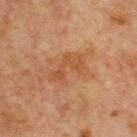This lesion was catalogued during total-body skin photography and was not selected for biopsy.
A male patient, about 65 years old.
Cropped from a total-body skin-imaging series; the visible field is about 15 mm.
The lesion's longest dimension is about 4.5 mm.
Captured under cross-polarized illumination.
From the chest.
An algorithmic analysis of the crop reported an eccentricity of roughly 0.8 and a shape-asymmetry score of about 0.5 (0 = symmetric).